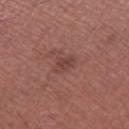No biopsy was performed on this lesion — it was imaged during a full skin examination and was not determined to be concerning.
A lesion tile, about 15 mm wide, cut from a 3D total-body photograph.
From the left thigh.
A male patient approximately 65 years of age.